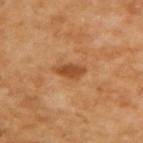Recorded during total-body skin imaging; not selected for excision or biopsy. Cropped from a total-body skin-imaging series; the visible field is about 15 mm. This is a cross-polarized tile. The subject is a male in their mid- to late 60s.